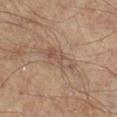Imaged during a routine full-body skin examination; the lesion was not biopsied and no histopathology is available. A 15 mm close-up tile from a total-body photography series done for melanoma screening. Located on the right lower leg. A patient roughly 55 years of age.